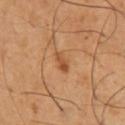The lesion was tiled from a total-body skin photograph and was not biopsied. Cropped from a total-body skin-imaging series; the visible field is about 15 mm. A male patient approximately 50 years of age. The lesion is located on the chest.